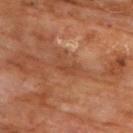| feature | finding |
|---|---|
| notes | total-body-photography surveillance lesion; no biopsy |
| site | the chest |
| diameter | ~3 mm (longest diameter) |
| automated lesion analysis | a footprint of about 4.5 mm², an eccentricity of roughly 0.85, and two-axis asymmetry of about 0.45; a border-irregularity rating of about 5.5/10, a within-lesion color-variation index near 1/10, and peripheral color asymmetry of about 0.5 |
| patient | male, approximately 60 years of age |
| acquisition | 15 mm crop, total-body photography |
| lighting | cross-polarized |